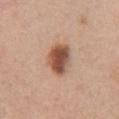{"biopsy_status": "not biopsied; imaged during a skin examination", "patient": {"sex": "female", "age_approx": 30}, "site": "chest", "lighting": "white-light", "automated_metrics": {"cielab_L": 52, "cielab_a": 22, "cielab_b": 30, "border_irregularity_0_10": 1.5, "color_variation_0_10": 6.5, "peripheral_color_asymmetry": 2.0, "nevus_likeness_0_100": 95, "lesion_detection_confidence_0_100": 100}, "image": {"source": "total-body photography crop", "field_of_view_mm": 15}}